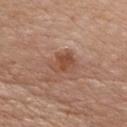Impression: Imaged during a routine full-body skin examination; the lesion was not biopsied and no histopathology is available. Background: The tile uses white-light illumination. From the chest. The subject is a male aged approximately 65. This image is a 15 mm lesion crop taken from a total-body photograph. About 3.5 mm across.